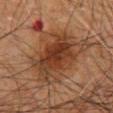workup = catalogued during a skin exam; not biopsied
image source = 15 mm crop, total-body photography
patient = male, aged approximately 60
location = the mid back